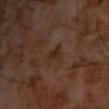This lesion was catalogued during total-body skin photography and was not selected for biopsy. Captured under cross-polarized illumination. A 15 mm close-up tile from a total-body photography series done for melanoma screening. A male subject, aged around 60. The lesion is on the chest.